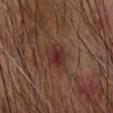<case>
<biopsy_status>not biopsied; imaged during a skin examination</biopsy_status>
<site>right forearm</site>
<automated_metrics>
  <cielab_L>31</cielab_L>
  <cielab_a>22</cielab_a>
  <cielab_b>23</cielab_b>
  <vs_skin_darker_L>7.0</vs_skin_darker_L>
  <vs_skin_contrast_norm>7.5</vs_skin_contrast_norm>
  <border_irregularity_0_10>2.0</border_irregularity_0_10>
  <peripheral_color_asymmetry>1.5</peripheral_color_asymmetry>
</automated_metrics>
<patient>
  <sex>male</sex>
  <age_approx>65</age_approx>
</patient>
<lesion_size>
  <long_diameter_mm_approx>3.0</long_diameter_mm_approx>
</lesion_size>
<image>
  <source>total-body photography crop</source>
  <field_of_view_mm>15</field_of_view_mm>
</image>
<lighting>cross-polarized</lighting>
</case>